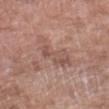Assessment:
Captured during whole-body skin photography for melanoma surveillance; the lesion was not biopsied.
Background:
A 15 mm close-up extracted from a 3D total-body photography capture. On the left forearm. A female subject, aged approximately 70.